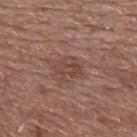<case>
  <biopsy_status>not biopsied; imaged during a skin examination</biopsy_status>
  <lesion_size>
    <long_diameter_mm_approx>3.0</long_diameter_mm_approx>
  </lesion_size>
  <site>upper back</site>
  <lighting>white-light</lighting>
  <image>
    <source>total-body photography crop</source>
    <field_of_view_mm>15</field_of_view_mm>
  </image>
  <patient>
    <sex>male</sex>
    <age_approx>60</age_approx>
  </patient>
</case>